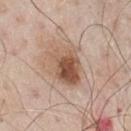workup: imaged on a skin check; not biopsied
anatomic site: the chest
subject: male, aged 78 to 82
image: ~15 mm crop, total-body skin-cancer survey